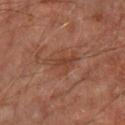Imaged during a routine full-body skin examination; the lesion was not biopsied and no histopathology is available. A 15 mm crop from a total-body photograph taken for skin-cancer surveillance. The total-body-photography lesion software estimated an area of roughly 6 mm², an outline eccentricity of about 0.8 (0 = round, 1 = elongated), and a symmetry-axis asymmetry near 0.45. And it measured a lesion color around L≈41 a*≈22 b*≈30 in CIELAB and a normalized lesion–skin contrast near 6. The software also gave a nevus-likeness score of about 0/100 and a lesion-detection confidence of about 100/100. A male patient, in their 70s. On the right lower leg. The lesion's longest dimension is about 4 mm. Captured under cross-polarized illumination.